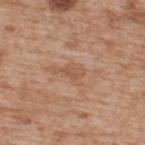Impression:
The lesion was photographed on a routine skin check and not biopsied; there is no pathology result.
Clinical summary:
A 15 mm close-up tile from a total-body photography series done for melanoma screening. From the upper back. The tile uses white-light illumination. Automated tile analysis of the lesion measured an average lesion color of about L≈54 a*≈21 b*≈32 (CIELAB) and a normalized border contrast of about 5. It also reported a border-irregularity index near 3.5/10 and peripheral color asymmetry of about 0.5. Approximately 2.5 mm at its widest. A male patient aged around 65.